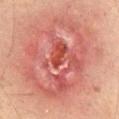  biopsy_status: not biopsied; imaged during a skin examination
  site: abdomen
  patient:
    sex: male
    age_approx: 60
  image:
    source: total-body photography crop
    field_of_view_mm: 15